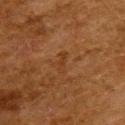notes=total-body-photography surveillance lesion; no biopsy
lesion diameter=about 3 mm
acquisition=total-body-photography crop, ~15 mm field of view
location=the upper back
patient=female, aged 48 to 52